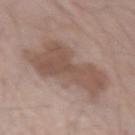Imaged during a routine full-body skin examination; the lesion was not biopsied and no histopathology is available.
A roughly 15 mm field-of-view crop from a total-body skin photograph.
The recorded lesion diameter is about 7.5 mm.
The subject is a male roughly 70 years of age.
Automated image analysis of the tile measured a border-irregularity index near 5.5/10, a within-lesion color-variation index near 2.5/10, and radial color variation of about 1. It also reported an automated nevus-likeness rating near 0 out of 100 and lesion-presence confidence of about 100/100.
From the left forearm.